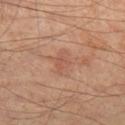The lesion was photographed on a routine skin check and not biopsied; there is no pathology result. The tile uses cross-polarized illumination. A male patient roughly 65 years of age. A lesion tile, about 15 mm wide, cut from a 3D total-body photograph. From the left thigh. About 2.5 mm across. The lesion-visualizer software estimated an automated nevus-likeness rating near 0 out of 100 and a lesion-detection confidence of about 100/100.